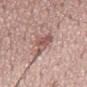Findings:
- follow-up · imaged on a skin check; not biopsied
- image · total-body-photography crop, ~15 mm field of view
- subject · male, in their mid-50s
- diameter · about 5 mm
- location · the mid back